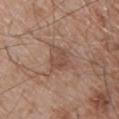Q: Was a biopsy performed?
A: total-body-photography surveillance lesion; no biopsy
Q: Who is the patient?
A: male, approximately 55 years of age
Q: What lighting was used for the tile?
A: white-light illumination
Q: Lesion location?
A: the upper back
Q: What did automated image analysis measure?
A: a footprint of about 5 mm², a shape eccentricity near 0.5, and two-axis asymmetry of about 0.3; border irregularity of about 3.5 on a 0–10 scale and a peripheral color-asymmetry measure near 0.5
Q: How was this image acquired?
A: 15 mm crop, total-body photography